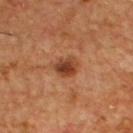<case>
  <biopsy_status>not biopsied; imaged during a skin examination</biopsy_status>
  <site>front of the torso</site>
  <image>
    <source>total-body photography crop</source>
    <field_of_view_mm>15</field_of_view_mm>
  </image>
  <patient>
    <sex>male</sex>
    <age_approx>55</age_approx>
  </patient>
  <lesion_size>
    <long_diameter_mm_approx>3.0</long_diameter_mm_approx>
  </lesion_size>
</case>